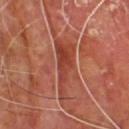Recorded during total-body skin imaging; not selected for excision or biopsy. A roughly 15 mm field-of-view crop from a total-body skin photograph. Approximately 7 mm at its widest. A male subject about 60 years old. Automated tile analysis of the lesion measured a within-lesion color-variation index near 4/10 and a peripheral color-asymmetry measure near 1.5. And it measured a lesion-detection confidence of about 80/100. From the upper back. Imaged with cross-polarized lighting.